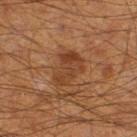follow-up: total-body-photography surveillance lesion; no biopsy | acquisition: ~15 mm crop, total-body skin-cancer survey | automated metrics: an area of roughly 9 mm², an eccentricity of roughly 0.8, and a shape-asymmetry score of about 0.35 (0 = symmetric); a border-irregularity index near 4/10, a color-variation rating of about 3.5/10, and radial color variation of about 1 | subject: male, in their 60s | lesion diameter: about 4.5 mm | anatomic site: the right thigh | lighting: cross-polarized illumination.